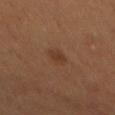• follow-up · no biopsy performed (imaged during a skin exam)
• lighting · cross-polarized
• location · the back
• image · total-body-photography crop, ~15 mm field of view
• patient · male, aged 53 to 57